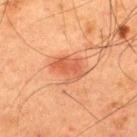| field | value |
|---|---|
| notes | catalogued during a skin exam; not biopsied |
| subject | male, aged around 60 |
| anatomic site | the upper back |
| image | ~15 mm crop, total-body skin-cancer survey |